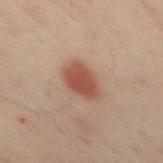patient = male, about 50 years old | location = the back | TBP lesion metrics = an eccentricity of roughly 0.75; a lesion color around L≈45 a*≈21 b*≈27 in CIELAB, a lesion–skin lightness drop of about 11, and a normalized border contrast of about 9; border irregularity of about 2 on a 0–10 scale, a color-variation rating of about 2.5/10, and a peripheral color-asymmetry measure near 0.5; a nevus-likeness score of about 100/100 and a detector confidence of about 100 out of 100 that the crop contains a lesion | lighting = cross-polarized illumination | image = 15 mm crop, total-body photography | diameter = ~4 mm (longest diameter).